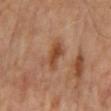This lesion was catalogued during total-body skin photography and was not selected for biopsy. The lesion is located on the mid back. A male subject aged 63–67. Imaged with cross-polarized lighting. The total-body-photography lesion software estimated an area of roughly 5 mm², an eccentricity of roughly 0.85, and two-axis asymmetry of about 0.3. It also reported a lesion color around L≈44 a*≈22 b*≈33 in CIELAB and roughly 10 lightness units darker than nearby skin. The software also gave an automated nevus-likeness rating near 10 out of 100 and a lesion-detection confidence of about 100/100. A region of skin cropped from a whole-body photographic capture, roughly 15 mm wide. Longest diameter approximately 3.5 mm.